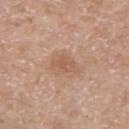image source = 15 mm crop, total-body photography
subject = male, about 55 years old
lighting = white-light illumination
lesion size = ≈3 mm
image-analysis metrics = a lesion color around L≈57 a*≈20 b*≈31 in CIELAB and a lesion–skin lightness drop of about 7; a border-irregularity rating of about 3/10 and peripheral color asymmetry of about 0.5; a classifier nevus-likeness of about 5/100 and lesion-presence confidence of about 100/100
body site = the right upper arm